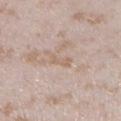Captured during whole-body skin photography for melanoma surveillance; the lesion was not biopsied. A lesion tile, about 15 mm wide, cut from a 3D total-body photograph. About 3 mm across. The lesion-visualizer software estimated about 6 CIELAB-L* units darker than the surrounding skin. The analysis additionally found a border-irregularity index near 4/10, a within-lesion color-variation index near 0/10, and peripheral color asymmetry of about 0. The software also gave a classifier nevus-likeness of about 0/100 and lesion-presence confidence of about 75/100. The lesion is on the leg. This is a white-light tile. The patient is a female in their mid- to late 20s.